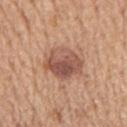This lesion was catalogued during total-body skin photography and was not selected for biopsy.
The recorded lesion diameter is about 4.5 mm.
A male patient in their mid- to late 60s.
The lesion is on the mid back.
Automated tile analysis of the lesion measured a classifier nevus-likeness of about 75/100.
A close-up tile cropped from a whole-body skin photograph, about 15 mm across.
The tile uses white-light illumination.